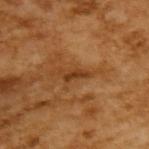Background: A male subject, aged approximately 65. A 15 mm crop from a total-body photograph taken for skin-cancer surveillance. Measured at roughly 3.5 mm in maximum diameter.